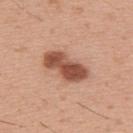No biopsy was performed on this lesion — it was imaged during a full skin examination and was not determined to be concerning. A male subject in their 30s. The recorded lesion diameter is about 5.5 mm. This image is a 15 mm lesion crop taken from a total-body photograph. This is a white-light tile. From the upper back.